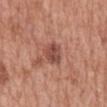follow-up: total-body-photography surveillance lesion; no biopsy | patient: male, aged around 70 | lesion size: ≈3.5 mm | site: the back | image: 15 mm crop, total-body photography.